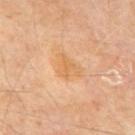Captured during whole-body skin photography for melanoma surveillance; the lesion was not biopsied. A 15 mm close-up tile from a total-body photography series done for melanoma screening. The lesion is located on the mid back. Captured under cross-polarized illumination. A male patient approximately 70 years of age.